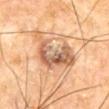Captured during whole-body skin photography for melanoma surveillance; the lesion was not biopsied.
Located on the chest.
The lesion's longest dimension is about 6.5 mm.
Captured under cross-polarized illumination.
Automated image analysis of the tile measured a footprint of about 17 mm², a shape eccentricity near 0.65, and a symmetry-axis asymmetry near 0.5. And it measured a lesion color around L≈59 a*≈20 b*≈33 in CIELAB, roughly 14 lightness units darker than nearby skin, and a normalized lesion–skin contrast near 9. And it measured a border-irregularity rating of about 7/10 and internal color variation of about 9 on a 0–10 scale. It also reported a classifier nevus-likeness of about 0/100 and a lesion-detection confidence of about 100/100.
Cropped from a whole-body photographic skin survey; the tile spans about 15 mm.
A male patient aged 63 to 67.